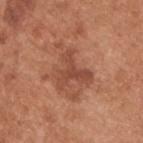<tbp_lesion>
  <biopsy_status>not biopsied; imaged during a skin examination</biopsy_status>
  <automated_metrics>
    <eccentricity>0.45</eccentricity>
    <shape_asymmetry>0.7</shape_asymmetry>
    <border_irregularity_0_10>8.0</border_irregularity_0_10>
    <peripheral_color_asymmetry>1.0</peripheral_color_asymmetry>
    <nevus_likeness_0_100>0</nevus_likeness_0_100>
    <lesion_detection_confidence_0_100>100</lesion_detection_confidence_0_100>
  </automated_metrics>
  <lighting>white-light</lighting>
  <site>upper back</site>
  <lesion_size>
    <long_diameter_mm_approx>4.5</long_diameter_mm_approx>
  </lesion_size>
  <patient>
    <sex>male</sex>
    <age_approx>55</age_approx>
  </patient>
  <image>
    <source>total-body photography crop</source>
    <field_of_view_mm>15</field_of_view_mm>
  </image>
</tbp_lesion>